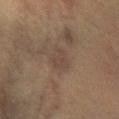lesion diameter=≈3 mm | automated metrics=an automated nevus-likeness rating near 0 out of 100 | illumination=cross-polarized illumination | location=the right lower leg | subject=female, aged approximately 30 | image=15 mm crop, total-body photography.